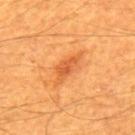<record>
  <biopsy_status>not biopsied; imaged during a skin examination</biopsy_status>
  <site>back</site>
  <patient>
    <sex>male</sex>
    <age_approx>60</age_approx>
  </patient>
  <image>
    <source>total-body photography crop</source>
    <field_of_view_mm>15</field_of_view_mm>
  </image>
</record>